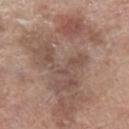The lesion was photographed on a routine skin check and not biopsied; there is no pathology result.
The total-body-photography lesion software estimated a lesion area of about 75 mm², an outline eccentricity of about 0.8 (0 = round, 1 = elongated), and a shape-asymmetry score of about 0.45 (0 = symmetric). The analysis additionally found a lesion color around L≈53 a*≈17 b*≈24 in CIELAB and a normalized border contrast of about 6.5. The software also gave border irregularity of about 7.5 on a 0–10 scale, a color-variation rating of about 6/10, and peripheral color asymmetry of about 2. It also reported an automated nevus-likeness rating near 0 out of 100 and a lesion-detection confidence of about 55/100.
The subject is a male in their 70s.
The lesion is located on the leg.
The tile uses white-light illumination.
Measured at roughly 15 mm in maximum diameter.
A region of skin cropped from a whole-body photographic capture, roughly 15 mm wide.